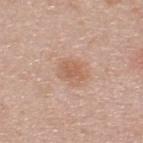biopsy status: total-body-photography surveillance lesion; no biopsy
automated metrics: a lesion color around L≈59 a*≈21 b*≈31 in CIELAB and a normalized border contrast of about 6; a nevus-likeness score of about 30/100 and a lesion-detection confidence of about 100/100
lesion size: ≈2.5 mm
site: the upper back
image source: ~15 mm tile from a whole-body skin photo
patient: male, aged approximately 45